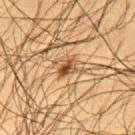The lesion is located on the upper back.
A 15 mm close-up extracted from a 3D total-body photography capture.
An algorithmic analysis of the crop reported an area of roughly 4 mm² and a shape-asymmetry score of about 0.25 (0 = symmetric). The analysis additionally found about 11 CIELAB-L* units darker than the surrounding skin. The software also gave internal color variation of about 7 on a 0–10 scale.
A male subject aged 58–62.
The tile uses cross-polarized illumination.